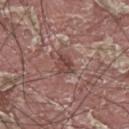Notes:
• subject: male, aged 38 to 42
• image: total-body-photography crop, ~15 mm field of view
• lesion diameter: ≈3 mm
• TBP lesion metrics: an average lesion color of about L≈43 a*≈21 b*≈22 (CIELAB) and a normalized lesion–skin contrast near 7.5; a border-irregularity index near 2.5/10, a within-lesion color-variation index near 4/10, and a peripheral color-asymmetry measure near 1.5; a classifier nevus-likeness of about 5/100 and a lesion-detection confidence of about 90/100
• tile lighting: white-light illumination
• site: the upper back